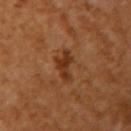| key | value |
|---|---|
| workup | total-body-photography surveillance lesion; no biopsy |
| automated metrics | a lesion area of about 5.5 mm², an eccentricity of roughly 0.8, and a symmetry-axis asymmetry near 0.45; a mean CIELAB color near L≈36 a*≈25 b*≈35, about 9 CIELAB-L* units darker than the surrounding skin, and a normalized border contrast of about 8; a border-irregularity index near 5/10 and internal color variation of about 2.5 on a 0–10 scale |
| image | ~15 mm tile from a whole-body skin photo |
| patient | female, roughly 55 years of age |
| size | ≈3.5 mm |
| anatomic site | the right upper arm |